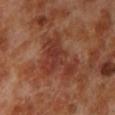The lesion was tiled from a total-body skin photograph and was not biopsied.
The tile uses cross-polarized illumination.
Automated tile analysis of the lesion measured an area of roughly 16 mm², an eccentricity of roughly 0.75, and a shape-asymmetry score of about 0.6 (0 = symmetric). The analysis additionally found a lesion color around L≈35 a*≈25 b*≈28 in CIELAB, a lesion–skin lightness drop of about 8, and a normalized lesion–skin contrast near 7. The analysis additionally found a border-irregularity index near 8/10, a within-lesion color-variation index near 4/10, and radial color variation of about 1.5. The analysis additionally found a classifier nevus-likeness of about 0/100 and a detector confidence of about 100 out of 100 that the crop contains a lesion.
Measured at roughly 6 mm in maximum diameter.
The patient is a male aged 68–72.
On the leg.
This image is a 15 mm lesion crop taken from a total-body photograph.